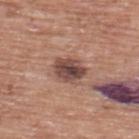Q: What are the patient's age and sex?
A: male, approximately 75 years of age
Q: Illumination type?
A: white-light illumination
Q: What did automated image analysis measure?
A: an outline eccentricity of about 0.65 (0 = round, 1 = elongated); a nevus-likeness score of about 20/100 and lesion-presence confidence of about 100/100
Q: Lesion location?
A: the back
Q: Lesion size?
A: ≈3.5 mm
Q: What is the imaging modality?
A: 15 mm crop, total-body photography